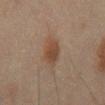Captured during whole-body skin photography for melanoma surveillance; the lesion was not biopsied.
A 15 mm close-up extracted from a 3D total-body photography capture.
The lesion is on the front of the torso.
This is a cross-polarized tile.
A male patient aged 43 to 47.
The lesion-visualizer software estimated a footprint of about 7 mm², an outline eccentricity of about 0.8 (0 = round, 1 = elongated), and two-axis asymmetry of about 0.2. The analysis additionally found about 7 CIELAB-L* units darker than the surrounding skin and a normalized border contrast of about 7.5. And it measured a nevus-likeness score of about 100/100 and lesion-presence confidence of about 100/100.
Measured at roughly 4 mm in maximum diameter.